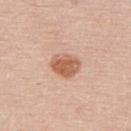{
  "site": "back",
  "patient": {
    "sex": "male",
    "age_approx": 60
  },
  "image": {
    "source": "total-body photography crop",
    "field_of_view_mm": 15
  },
  "lesion_size": {
    "long_diameter_mm_approx": 3.5
  },
  "lighting": "white-light"
}